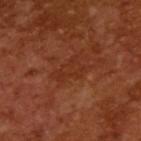Imaged with cross-polarized lighting. A male subject aged 63–67. A 15 mm crop from a total-body photograph taken for skin-cancer surveillance. Automated image analysis of the tile measured an area of roughly 6.5 mm² and an eccentricity of roughly 0.8. And it measured a border-irregularity index near 5/10, a within-lesion color-variation index near 1.5/10, and a peripheral color-asymmetry measure near 0.5. The software also gave an automated nevus-likeness rating near 0 out of 100. Longest diameter approximately 3.5 mm.